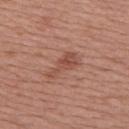- biopsy status: no biopsy performed (imaged during a skin exam)
- location: the upper back
- acquisition: 15 mm crop, total-body photography
- TBP lesion metrics: a shape eccentricity near 0.9; a mean CIELAB color near L≈49 a*≈24 b*≈28 and a normalized lesion–skin contrast near 6.5; a border-irregularity index near 6.5/10, a within-lesion color-variation index near 3/10, and a peripheral color-asymmetry measure near 1; a nevus-likeness score of about 0/100
- tile lighting: white-light illumination
- size: about 4 mm
- patient: female, roughly 50 years of age